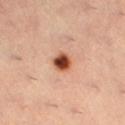This lesion was catalogued during total-body skin photography and was not selected for biopsy.